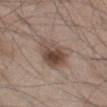The patient is a male aged approximately 45. Measured at roughly 5 mm in maximum diameter. This is a white-light tile. Located on the left thigh. Cropped from a whole-body photographic skin survey; the tile spans about 15 mm.